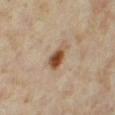Case summary:
- follow-up — total-body-photography surveillance lesion; no biopsy
- image — ~15 mm tile from a whole-body skin photo
- subject — female, approximately 35 years of age
- site — the left thigh
- diameter — about 3.5 mm
- tile lighting — cross-polarized